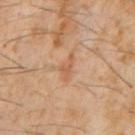Case summary:
• follow-up · catalogued during a skin exam; not biopsied
• acquisition · ~15 mm tile from a whole-body skin photo
• location · the chest
• subject · male, aged approximately 60
• illumination · cross-polarized illumination